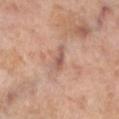Part of a total-body skin-imaging series; this lesion was reviewed on a skin check and was not flagged for biopsy.
About 3.5 mm across.
From the left lower leg.
This is a cross-polarized tile.
Automated tile analysis of the lesion measured a footprint of about 3 mm², an outline eccentricity of about 0.95 (0 = round, 1 = elongated), and a symmetry-axis asymmetry near 0.35. And it measured an average lesion color of about L≈56 a*≈22 b*≈26 (CIELAB), about 10 CIELAB-L* units darker than the surrounding skin, and a lesion-to-skin contrast of about 7 (normalized; higher = more distinct). It also reported lesion-presence confidence of about 75/100.
A close-up tile cropped from a whole-body skin photograph, about 15 mm across.
The patient is a female roughly 55 years of age.